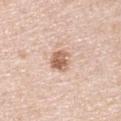Case summary:
– imaging modality · 15 mm crop, total-body photography
– body site · the right upper arm
– subject · male, aged approximately 50